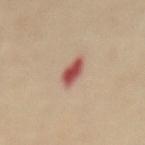Impression: Imaged during a routine full-body skin examination; the lesion was not biopsied and no histopathology is available. Image and clinical context: Captured under cross-polarized illumination. On the front of the torso. A female patient, about 70 years old. Cropped from a total-body skin-imaging series; the visible field is about 15 mm.